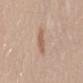No biopsy was performed on this lesion — it was imaged during a full skin examination and was not determined to be concerning. Captured under white-light illumination. A female subject approximately 30 years of age. The lesion's longest dimension is about 3.5 mm. The lesion is located on the lower back. A 15 mm close-up extracted from a 3D total-body photography capture.